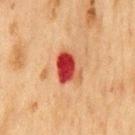Cropped from a total-body skin-imaging series; the visible field is about 15 mm. The lesion is located on the chest. Captured under cross-polarized illumination. A male patient aged approximately 75. The recorded lesion diameter is about 4 mm.